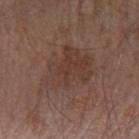Q: Is there a histopathology result?
A: total-body-photography surveillance lesion; no biopsy
Q: Automated lesion metrics?
A: roughly 6 lightness units darker than nearby skin and a normalized border contrast of about 5.5; internal color variation of about 3.5 on a 0–10 scale and radial color variation of about 1; a nevus-likeness score of about 0/100 and lesion-presence confidence of about 100/100
Q: How was this image acquired?
A: ~15 mm tile from a whole-body skin photo
Q: What is the anatomic site?
A: the left forearm
Q: How large is the lesion?
A: about 7 mm
Q: Who is the patient?
A: male, roughly 55 years of age